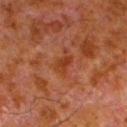| field | value |
|---|---|
| biopsy status | total-body-photography surveillance lesion; no biopsy |
| body site | the left lower leg |
| lesion size | ~2.5 mm (longest diameter) |
| patient | male, aged 78 to 82 |
| image source | ~15 mm tile from a whole-body skin photo |